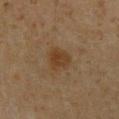Clinical impression:
Part of a total-body skin-imaging series; this lesion was reviewed on a skin check and was not flagged for biopsy.
Acquisition and patient details:
A 15 mm close-up extracted from a 3D total-body photography capture. The tile uses cross-polarized illumination. Located on the front of the torso. About 3 mm across. An algorithmic analysis of the crop reported a lesion color around L≈31 a*≈15 b*≈27 in CIELAB, roughly 6 lightness units darker than nearby skin, and a normalized border contrast of about 7. It also reported lesion-presence confidence of about 100/100. A male subject aged approximately 60.